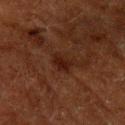– workup: imaged on a skin check; not biopsied
– size: ~3 mm (longest diameter)
– illumination: cross-polarized
– image: ~15 mm crop, total-body skin-cancer survey
– automated lesion analysis: a lesion area of about 3.5 mm² and an eccentricity of roughly 0.8; about 6 CIELAB-L* units darker than the surrounding skin and a normalized border contrast of about 8
– subject: male, aged around 60
– body site: the left upper arm